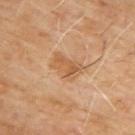follow-up: imaged on a skin check; not biopsied
automated lesion analysis: an outline eccentricity of about 0.8 (0 = round, 1 = elongated) and a shape-asymmetry score of about 0.25 (0 = symmetric); a mean CIELAB color near L≈55 a*≈20 b*≈36 and a normalized lesion–skin contrast near 6; a border-irregularity index near 3/10, a within-lesion color-variation index near 3.5/10, and a peripheral color-asymmetry measure near 1.5
patient: male, aged 63–67
illumination: cross-polarized illumination
lesion size: ≈4 mm
location: the upper back
image source: total-body-photography crop, ~15 mm field of view